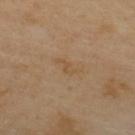The lesion was tiled from a total-body skin photograph and was not biopsied. This is a cross-polarized tile. The lesion's longest dimension is about 3 mm. Cropped from a whole-body photographic skin survey; the tile spans about 15 mm. A male patient, aged around 55. Located on the upper back.